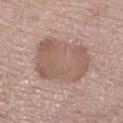  lighting: white-light
  site: left lower leg
  lesion_size:
    long_diameter_mm_approx: 6.5
  automated_metrics:
    area_mm2_approx: 30.0
    eccentricity: 0.45
    shape_asymmetry: 0.1
  image:
    source: total-body photography crop
    field_of_view_mm: 15
  patient:
    sex: female
    age_approx: 75